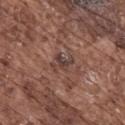Imaged during a routine full-body skin examination; the lesion was not biopsied and no histopathology is available. Imaged with white-light lighting. On the back. Measured at roughly 3 mm in maximum diameter. A 15 mm close-up tile from a total-body photography series done for melanoma screening. A male patient, in their mid-70s.